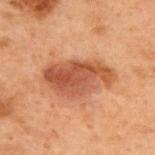| field | value |
|---|---|
| notes | imaged on a skin check; not biopsied |
| site | the upper back |
| lesion diameter | ~7.5 mm (longest diameter) |
| tile lighting | cross-polarized illumination |
| patient | male, aged around 70 |
| image | total-body-photography crop, ~15 mm field of view |